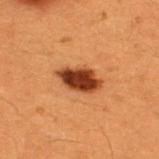Part of a total-body skin-imaging series; this lesion was reviewed on a skin check and was not flagged for biopsy. The patient is a male roughly 50 years of age. The lesion is located on the upper back. This image is a 15 mm lesion crop taken from a total-body photograph.